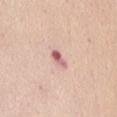- notes — catalogued during a skin exam; not biopsied
- tile lighting — white-light
- acquisition — total-body-photography crop, ~15 mm field of view
- patient — female, aged 63 to 67
- lesion size — about 2.5 mm
- site — the abdomen